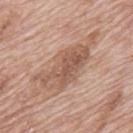Imaged during a routine full-body skin examination; the lesion was not biopsied and no histopathology is available. About 9.5 mm across. The lesion is located on the mid back. Cropped from a total-body skin-imaging series; the visible field is about 15 mm. A male subject, aged 68 to 72. The lesion-visualizer software estimated a lesion area of about 22 mm², an outline eccentricity of about 0.9 (0 = round, 1 = elongated), and a symmetry-axis asymmetry near 0.4. And it measured border irregularity of about 6.5 on a 0–10 scale, a color-variation rating of about 5/10, and peripheral color asymmetry of about 2. This is a white-light tile.